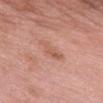Located on the front of the torso. Captured under white-light illumination. Measured at roughly 4 mm in maximum diameter. A roughly 15 mm field-of-view crop from a total-body skin photograph. A female subject, roughly 70 years of age.